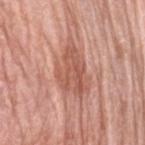illumination: white-light
automated metrics: border irregularity of about 4.5 on a 0–10 scale, a color-variation rating of about 3.5/10, and peripheral color asymmetry of about 1.5; a nevus-likeness score of about 0/100 and a detector confidence of about 100 out of 100 that the crop contains a lesion
subject: female, aged 58–62
body site: the left upper arm
image: total-body-photography crop, ~15 mm field of view